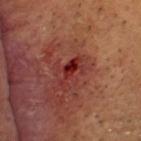Imaged during a routine full-body skin examination; the lesion was not biopsied and no histopathology is available.
Imaged with cross-polarized lighting.
Approximately 3.5 mm at its widest.
The lesion-visualizer software estimated a lesion area of about 5 mm², an eccentricity of roughly 0.9, and a symmetry-axis asymmetry near 0.4. It also reported an average lesion color of about L≈32 a*≈34 b*≈27 (CIELAB), roughly 10 lightness units darker than nearby skin, and a normalized lesion–skin contrast near 9. The analysis additionally found a detector confidence of about 100 out of 100 that the crop contains a lesion.
A region of skin cropped from a whole-body photographic capture, roughly 15 mm wide.
The patient is a male approximately 65 years of age.
On the head or neck.